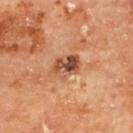Q: Is there a histopathology result?
A: no biopsy performed (imaged during a skin exam)
Q: What lighting was used for the tile?
A: cross-polarized
Q: How was this image acquired?
A: ~15 mm crop, total-body skin-cancer survey
Q: What are the patient's age and sex?
A: male, aged 63–67
Q: What is the lesion's diameter?
A: about 3.5 mm
Q: Lesion location?
A: the upper back